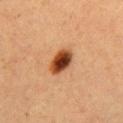Assessment: Captured during whole-body skin photography for melanoma surveillance; the lesion was not biopsied. Context: A 15 mm close-up tile from a total-body photography series done for melanoma screening. Captured under cross-polarized illumination. Automated image analysis of the tile measured a lesion area of about 7.5 mm², an eccentricity of roughly 0.8, and a shape-asymmetry score of about 0.15 (0 = symmetric). It also reported about 17 CIELAB-L* units darker than the surrounding skin and a normalized lesion–skin contrast near 13.5. The analysis additionally found border irregularity of about 1.5 on a 0–10 scale. The patient is a female roughly 40 years of age. The lesion's longest dimension is about 4 mm. Located on the chest.